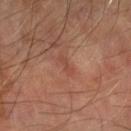Case summary:
• workup — no biopsy performed (imaged during a skin exam)
• illumination — cross-polarized illumination
• location — the left forearm
• acquisition — ~15 mm tile from a whole-body skin photo
• diameter — ≈2.5 mm
• subject — male, aged 68 to 72